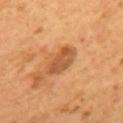This lesion was catalogued during total-body skin photography and was not selected for biopsy.